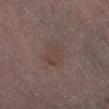Notes:
- notes: imaged on a skin check; not biopsied
- imaging modality: ~15 mm crop, total-body skin-cancer survey
- subject: female, in their 80s
- image-analysis metrics: a footprint of about 8 mm², an eccentricity of roughly 0.7, and two-axis asymmetry of about 0.25; a lesion color around L≈36 a*≈14 b*≈17 in CIELAB, roughly 4 lightness units darker than nearby skin, and a normalized border contrast of about 5; a border-irregularity rating of about 2.5/10 and internal color variation of about 2 on a 0–10 scale; a classifier nevus-likeness of about 0/100 and lesion-presence confidence of about 100/100
- lesion size: ~3.5 mm (longest diameter)
- site: the right leg